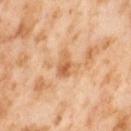Assessment: No biopsy was performed on this lesion — it was imaged during a full skin examination and was not determined to be concerning. Background: Approximately 3 mm at its widest. A female subject, in their mid-50s. A roughly 15 mm field-of-view crop from a total-body skin photograph. Located on the right thigh.